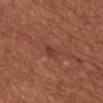<tbp_lesion>
  <biopsy_status>not biopsied; imaged during a skin examination</biopsy_status>
  <site>chest</site>
  <patient>
    <sex>female</sex>
    <age_approx>65</age_approx>
  </patient>
  <image>
    <source>total-body photography crop</source>
    <field_of_view_mm>15</field_of_view_mm>
  </image>
  <lighting>white-light</lighting>
  <lesion_size>
    <long_diameter_mm_approx>3.0</long_diameter_mm_approx>
  </lesion_size>
  <automated_metrics>
    <vs_skin_darker_L>7.0</vs_skin_darker_L>
    <vs_skin_contrast_norm>6.5</vs_skin_contrast_norm>
    <nevus_likeness_0_100>0</nevus_likeness_0_100>
    <lesion_detection_confidence_0_100>100</lesion_detection_confidence_0_100>
  </automated_metrics>
</tbp_lesion>